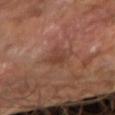The lesion was tiled from a total-body skin photograph and was not biopsied.
On the arm.
The subject is a male aged approximately 60.
This is a cross-polarized tile.
Approximately 3 mm at its widest.
A 15 mm close-up extracted from a 3D total-body photography capture.